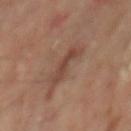notes — total-body-photography surveillance lesion; no biopsy
TBP lesion metrics — a footprint of about 7.5 mm², an eccentricity of roughly 0.9, and a shape-asymmetry score of about 0.35 (0 = symmetric); a mean CIELAB color near L≈43 a*≈19 b*≈26, a lesion–skin lightness drop of about 8, and a normalized border contrast of about 6; a border-irregularity index near 4.5/10, a within-lesion color-variation index near 3/10, and peripheral color asymmetry of about 1; an automated nevus-likeness rating near 0 out of 100 and lesion-presence confidence of about 100/100
patient — male, approximately 65 years of age
image source — ~15 mm crop, total-body skin-cancer survey
size — ≈4.5 mm
tile lighting — cross-polarized
anatomic site — the mid back